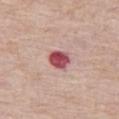notes = imaged on a skin check; not biopsied | subject = female, approximately 60 years of age | automated metrics = a lesion area of about 6 mm² and two-axis asymmetry of about 0.15 | lesion diameter = ~2.5 mm (longest diameter) | acquisition = 15 mm crop, total-body photography | illumination = white-light illumination | site = the left thigh.